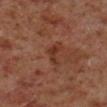<case>
<biopsy_status>not biopsied; imaged during a skin examination</biopsy_status>
<patient>
  <sex>male</sex>
  <age_approx>60</age_approx>
</patient>
<image>
  <source>total-body photography crop</source>
  <field_of_view_mm>15</field_of_view_mm>
</image>
<lighting>cross-polarized</lighting>
<site>right lower leg</site>
<lesion_size>
  <long_diameter_mm_approx>2.5</long_diameter_mm_approx>
</lesion_size>
</case>